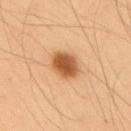The lesion was photographed on a routine skin check and not biopsied; there is no pathology result. An algorithmic analysis of the crop reported a border-irregularity index near 1.5/10 and a color-variation rating of about 4/10. It also reported an automated nevus-likeness rating near 100 out of 100 and lesion-presence confidence of about 100/100. A 15 mm close-up extracted from a 3D total-body photography capture. About 4 mm across. The lesion is on the upper back. A male subject, approximately 55 years of age.